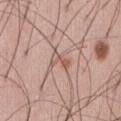biopsy_status: not biopsied; imaged during a skin examination
lighting: white-light
patient:
  sex: male
  age_approx: 65
automated_metrics:
  cielab_L: 57
  cielab_a: 19
  cielab_b: 26
  vs_skin_darker_L: 9.0
  border_irregularity_0_10: 5.5
  color_variation_0_10: 0.0
  peripheral_color_asymmetry: 0.0
  lesion_detection_confidence_0_100: 90
site: front of the torso
image:
  source: total-body photography crop
  field_of_view_mm: 15
lesion_size:
  long_diameter_mm_approx: 2.5